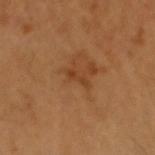A 15 mm close-up tile from a total-body photography series done for melanoma screening. The lesion is located on the head or neck. A male patient, aged 43 to 47.